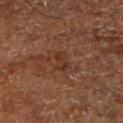Assessment:
Recorded during total-body skin imaging; not selected for excision or biopsy.
Clinical summary:
A subject approximately 65 years of age. The total-body-photography lesion software estimated a lesion area of about 3.5 mm², a shape eccentricity near 0.85, and a symmetry-axis asymmetry near 0.3. And it measured a border-irregularity rating of about 3.5/10 and a color-variation rating of about 1/10. From the right lower leg. A roughly 15 mm field-of-view crop from a total-body skin photograph.